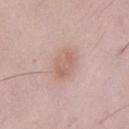Image and clinical context:
Automated tile analysis of the lesion measured a lesion area of about 8 mm², an outline eccentricity of about 0.8 (0 = round, 1 = elongated), and a shape-asymmetry score of about 0.1 (0 = symmetric). It also reported a lesion color around L≈62 a*≈19 b*≈27 in CIELAB, roughly 8 lightness units darker than nearby skin, and a normalized border contrast of about 6. The software also gave a border-irregularity index near 1.5/10, internal color variation of about 3 on a 0–10 scale, and radial color variation of about 1. It also reported a lesion-detection confidence of about 100/100. A male subject, aged around 50. Located on the chest. Captured under white-light illumination. The recorded lesion diameter is about 4 mm. This image is a 15 mm lesion crop taken from a total-body photograph.